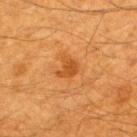Case summary:
* workup: no biopsy performed (imaged during a skin exam)
* site: the upper back
* imaging modality: total-body-photography crop, ~15 mm field of view
* patient: male, aged around 60